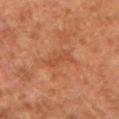Findings:
• biopsy status: total-body-photography surveillance lesion; no biopsy
• location: the arm
• TBP lesion metrics: a footprint of about 3 mm², an outline eccentricity of about 0.95 (0 = round, 1 = elongated), and a symmetry-axis asymmetry near 0.3
• subject: male, approximately 55 years of age
• lesion diameter: about 3.5 mm
• illumination: cross-polarized illumination
• image: ~15 mm crop, total-body skin-cancer survey